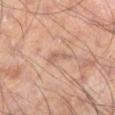The lesion was tiled from a total-body skin photograph and was not biopsied. A 15 mm crop from a total-body photograph taken for skin-cancer surveillance. About 2.5 mm across. The lesion-visualizer software estimated a lesion area of about 2.5 mm², an eccentricity of roughly 0.9, and two-axis asymmetry of about 0.45. The subject is a male about 55 years old. Imaged with cross-polarized lighting. On the leg.